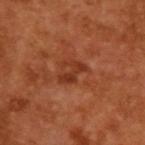biopsy status — no biopsy performed (imaged during a skin exam) | acquisition — total-body-photography crop, ~15 mm field of view | patient — male, roughly 65 years of age.